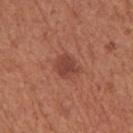TBP lesion metrics: a classifier nevus-likeness of about 85/100 and lesion-presence confidence of about 100/100; subject: female, in their mid-60s; location: the right upper arm; image source: 15 mm crop, total-body photography.